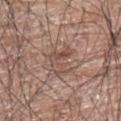The lesion was photographed on a routine skin check and not biopsied; there is no pathology result.
Measured at roughly 4 mm in maximum diameter.
A male subject aged approximately 70.
A lesion tile, about 15 mm wide, cut from a 3D total-body photograph.
This is a white-light tile.
The lesion is on the left upper arm.
An algorithmic analysis of the crop reported a classifier nevus-likeness of about 0/100.